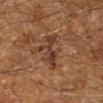The lesion was photographed on a routine skin check and not biopsied; there is no pathology result. Longest diameter approximately 5 mm. A male patient, roughly 60 years of age. This image is a 15 mm lesion crop taken from a total-body photograph. This is a cross-polarized tile. The lesion is located on the right lower leg.